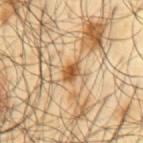Assessment:
Captured during whole-body skin photography for melanoma surveillance; the lesion was not biopsied.
Clinical summary:
About 2.5 mm across. Cropped from a whole-body photographic skin survey; the tile spans about 15 mm. On the mid back. A male patient, aged approximately 65.